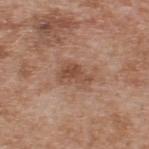Q: Was this lesion biopsied?
A: total-body-photography surveillance lesion; no biopsy
Q: How was this image acquired?
A: 15 mm crop, total-body photography
Q: Patient demographics?
A: male, aged approximately 65
Q: Where on the body is the lesion?
A: the upper back
Q: How was the tile lit?
A: white-light illumination